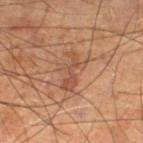follow-up: imaged on a skin check; not biopsied
image-analysis metrics: an area of roughly 8 mm², a shape eccentricity near 0.85, and a symmetry-axis asymmetry near 0.55; border irregularity of about 8.5 on a 0–10 scale and peripheral color asymmetry of about 0.5
acquisition: ~15 mm tile from a whole-body skin photo
subject: male, aged 68 to 72
illumination: cross-polarized
lesion size: ~4.5 mm (longest diameter)
body site: the left lower leg